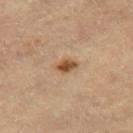<tbp_lesion>
<biopsy_status>not biopsied; imaged during a skin examination</biopsy_status>
</tbp_lesion>